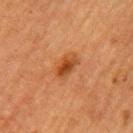Assessment:
Captured during whole-body skin photography for melanoma surveillance; the lesion was not biopsied.
Clinical summary:
The patient is a female in their mid-50s. The recorded lesion diameter is about 3.5 mm. Imaged with cross-polarized lighting. On the left upper arm. A region of skin cropped from a whole-body photographic capture, roughly 15 mm wide.